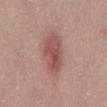Findings:
– biopsy status · imaged on a skin check; not biopsied
– lesion diameter · about 5 mm
– patient · male, in their mid-40s
– lighting · white-light
– site · the mid back
– TBP lesion metrics · an area of roughly 12 mm², an eccentricity of roughly 0.8, and a shape-asymmetry score of about 0.2 (0 = symmetric); a border-irregularity index near 2.5/10, internal color variation of about 4 on a 0–10 scale, and peripheral color asymmetry of about 1.5; an automated nevus-likeness rating near 95 out of 100 and lesion-presence confidence of about 100/100
– acquisition · ~15 mm crop, total-body skin-cancer survey